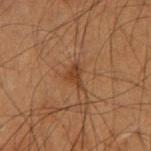This lesion was catalogued during total-body skin photography and was not selected for biopsy. The lesion is on the right forearm. A roughly 15 mm field-of-view crop from a total-body skin photograph. The subject is a male roughly 55 years of age.